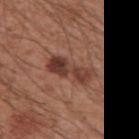– follow-up — no biopsy performed (imaged during a skin exam)
– tile lighting — white-light illumination
– anatomic site — the right upper arm
– TBP lesion metrics — a shape eccentricity near 0.95 and a shape-asymmetry score of about 0.25 (0 = symmetric); roughly 12 lightness units darker than nearby skin; border irregularity of about 4 on a 0–10 scale, a within-lesion color-variation index near 6/10, and radial color variation of about 2
– acquisition — ~15 mm tile from a whole-body skin photo
– subject — male, approximately 60 years of age